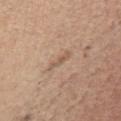Clinical impression:
Recorded during total-body skin imaging; not selected for excision or biopsy.
Background:
The tile uses white-light illumination. This image is a 15 mm lesion crop taken from a total-body photograph. Automated tile analysis of the lesion measured a footprint of about 2.5 mm², a shape eccentricity near 0.95, and a shape-asymmetry score of about 0.3 (0 = symmetric). And it measured a lesion color around L≈57 a*≈17 b*≈29 in CIELAB, about 7 CIELAB-L* units darker than the surrounding skin, and a normalized lesion–skin contrast near 5. The analysis additionally found internal color variation of about 0 on a 0–10 scale. And it measured a classifier nevus-likeness of about 0/100. From the chest. The lesion's longest dimension is about 3 mm. The subject is a female about 60 years old.